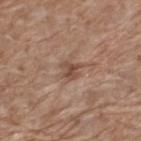Clinical impression:
Recorded during total-body skin imaging; not selected for excision or biopsy.
Clinical summary:
The lesion-visualizer software estimated an area of roughly 4.5 mm², an outline eccentricity of about 0.55 (0 = round, 1 = elongated), and a symmetry-axis asymmetry near 0.25. The lesion's longest dimension is about 2.5 mm. From the upper back. The subject is a male approximately 70 years of age. A 15 mm close-up tile from a total-body photography series done for melanoma screening. This is a white-light tile.